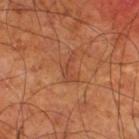biopsy status: total-body-photography surveillance lesion; no biopsy | automated metrics: a shape eccentricity near 0.9 and two-axis asymmetry of about 0.65; a lesion color around L≈44 a*≈25 b*≈33 in CIELAB; internal color variation of about 0 on a 0–10 scale and radial color variation of about 0 | illumination: cross-polarized illumination | size: about 3.5 mm | patient: male, roughly 70 years of age | imaging modality: ~15 mm crop, total-body skin-cancer survey | site: the right thigh.